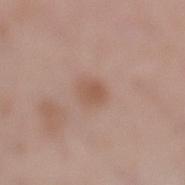  biopsy_status: not biopsied; imaged during a skin examination
  image:
    source: total-body photography crop
    field_of_view_mm: 15
  lesion_size:
    long_diameter_mm_approx: 2.5
  site: left lower leg
  lighting: white-light
  patient:
    sex: female
    age_approx: 50